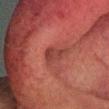<lesion>
  <biopsy_status>not biopsied; imaged during a skin examination</biopsy_status>
  <automated_metrics>
    <eccentricity>0.8</eccentricity>
    <shape_asymmetry>0.4</shape_asymmetry>
    <vs_skin_darker_L>7.0</vs_skin_darker_L>
    <vs_skin_contrast_norm>6.5</vs_skin_contrast_norm>
    <border_irregularity_0_10>4.0</border_irregularity_0_10>
    <color_variation_0_10>2.5</color_variation_0_10>
    <peripheral_color_asymmetry>0.5</peripheral_color_asymmetry>
    <nevus_likeness_0_100>0</nevus_likeness_0_100>
    <lesion_detection_confidence_0_100>80</lesion_detection_confidence_0_100>
  </automated_metrics>
  <lesion_size>
    <long_diameter_mm_approx>3.0</long_diameter_mm_approx>
  </lesion_size>
  <patient>
    <sex>male</sex>
    <age_approx>60</age_approx>
  </patient>
  <site>head or neck</site>
  <lighting>cross-polarized</lighting>
  <image>
    <source>total-body photography crop</source>
    <field_of_view_mm>15</field_of_view_mm>
  </image>
</lesion>